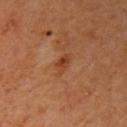Clinical impression:
The lesion was tiled from a total-body skin photograph and was not biopsied.
Acquisition and patient details:
Approximately 2.5 mm at its widest. The lesion-visualizer software estimated an area of roughly 3.5 mm², an outline eccentricity of about 0.8 (0 = round, 1 = elongated), and a shape-asymmetry score of about 0.25 (0 = symmetric). The analysis additionally found a border-irregularity index near 2.5/10, a within-lesion color-variation index near 3.5/10, and a peripheral color-asymmetry measure near 1. Imaged with cross-polarized lighting. On the right upper arm. A female subject, about 40 years old. A 15 mm close-up extracted from a 3D total-body photography capture.